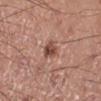biopsy_status: not biopsied; imaged during a skin examination
image:
  source: total-body photography crop
  field_of_view_mm: 15
patient:
  sex: male
  age_approx: 60
site: left lower leg